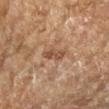Clinical impression: Recorded during total-body skin imaging; not selected for excision or biopsy. Background: The lesion-visualizer software estimated an area of roughly 3.5 mm². It also reported a mean CIELAB color near L≈47 a*≈20 b*≈29 and a lesion-to-skin contrast of about 6.5 (normalized; higher = more distinct). And it measured a border-irregularity index near 3.5/10 and radial color variation of about 1. And it measured a nevus-likeness score of about 0/100 and a lesion-detection confidence of about 100/100. The tile uses cross-polarized illumination. The lesion is on the right forearm. A region of skin cropped from a whole-body photographic capture, roughly 15 mm wide. Measured at roughly 2.5 mm in maximum diameter. The patient is a female aged approximately 70.